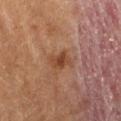The lesion was photographed on a routine skin check and not biopsied; there is no pathology result. This is a cross-polarized tile. A male patient, in their mid-80s or older. On the lower back. Cropped from a total-body skin-imaging series; the visible field is about 15 mm.